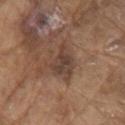Q: Was a biopsy performed?
A: catalogued during a skin exam; not biopsied
Q: Where on the body is the lesion?
A: the right upper arm
Q: What is the imaging modality?
A: ~15 mm tile from a whole-body skin photo
Q: Who is the patient?
A: male, in their 80s